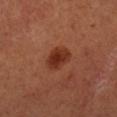workup: catalogued during a skin exam; not biopsied | site: the leg | TBP lesion metrics: a lesion area of about 6.5 mm², an outline eccentricity of about 0.75 (0 = round, 1 = elongated), and a shape-asymmetry score of about 0.25 (0 = symmetric) | patient: male, roughly 50 years of age | image source: ~15 mm crop, total-body skin-cancer survey | lesion size: about 3.5 mm.